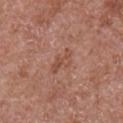This lesion was catalogued during total-body skin photography and was not selected for biopsy. The total-body-photography lesion software estimated an area of roughly 4 mm², an outline eccentricity of about 0.9 (0 = round, 1 = elongated), and a shape-asymmetry score of about 0.4 (0 = symmetric). The software also gave a lesion color around L≈49 a*≈23 b*≈29 in CIELAB and a lesion–skin lightness drop of about 7. The analysis additionally found internal color variation of about 0 on a 0–10 scale and peripheral color asymmetry of about 0. A male subject, about 65 years old. The lesion is located on the right upper arm. A region of skin cropped from a whole-body photographic capture, roughly 15 mm wide. Measured at roughly 3.5 mm in maximum diameter.